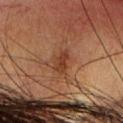Case summary:
* biopsy status: no biopsy performed (imaged during a skin exam)
* location: the head or neck
* TBP lesion metrics: an outline eccentricity of about 0.75 (0 = round, 1 = elongated) and a symmetry-axis asymmetry near 0.2; a nevus-likeness score of about 15/100 and a detector confidence of about 100 out of 100 that the crop contains a lesion
* image: ~15 mm crop, total-body skin-cancer survey
* tile lighting: cross-polarized
* size: ≈3 mm
* subject: female, aged approximately 40